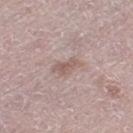<tbp_lesion>
  <biopsy_status>not biopsied; imaged during a skin examination</biopsy_status>
  <automated_metrics>
    <area_mm2_approx>4.5</area_mm2_approx>
    <eccentricity>0.9</eccentricity>
    <shape_asymmetry>0.35</shape_asymmetry>
    <cielab_L>57</cielab_L>
    <cielab_a>16</cielab_a>
    <cielab_b>21</cielab_b>
    <vs_skin_darker_L>8.0</vs_skin_darker_L>
    <vs_skin_contrast_norm>6.0</vs_skin_contrast_norm>
    <border_irregularity_0_10>3.5</border_irregularity_0_10>
    <color_variation_0_10>1.5</color_variation_0_10>
    <peripheral_color_asymmetry>0.5</peripheral_color_asymmetry>
  </automated_metrics>
  <image>
    <source>total-body photography crop</source>
    <field_of_view_mm>15</field_of_view_mm>
  </image>
  <patient>
    <sex>female</sex>
    <age_approx>65</age_approx>
  </patient>
  <lesion_size>
    <long_diameter_mm_approx>3.5</long_diameter_mm_approx>
  </lesion_size>
  <site>left thigh</site>
</tbp_lesion>